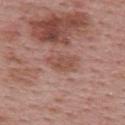Part of a total-body skin-imaging series; this lesion was reviewed on a skin check and was not flagged for biopsy.
A female subject, aged 53 to 57.
A lesion tile, about 15 mm wide, cut from a 3D total-body photograph.
On the upper back.